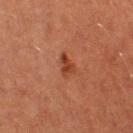Q: Was a biopsy performed?
A: imaged on a skin check; not biopsied
Q: What is the anatomic site?
A: the left thigh
Q: How large is the lesion?
A: about 2.5 mm
Q: What is the imaging modality?
A: ~15 mm crop, total-body skin-cancer survey
Q: Patient demographics?
A: female, aged 38–42
Q: Automated lesion metrics?
A: lesion-presence confidence of about 100/100
Q: What lighting was used for the tile?
A: cross-polarized